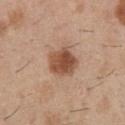Notes:
* notes — imaged on a skin check; not biopsied
* patient — male, approximately 30 years of age
* location — the front of the torso
* image — 15 mm crop, total-body photography
* diameter — about 3.5 mm
* TBP lesion metrics — lesion-presence confidence of about 100/100